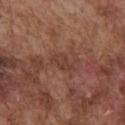Q: Was this lesion biopsied?
A: catalogued during a skin exam; not biopsied
Q: Where on the body is the lesion?
A: the chest
Q: How was this image acquired?
A: ~15 mm crop, total-body skin-cancer survey
Q: What lighting was used for the tile?
A: white-light
Q: Lesion size?
A: ~3 mm (longest diameter)
Q: Automated lesion metrics?
A: a lesion area of about 4.5 mm² and a shape-asymmetry score of about 0.3 (0 = symmetric); a mean CIELAB color near L≈40 a*≈21 b*≈27, roughly 6 lightness units darker than nearby skin, and a normalized border contrast of about 5.5; border irregularity of about 3.5 on a 0–10 scale and radial color variation of about 0.5
Q: Patient demographics?
A: male, in their mid- to late 70s